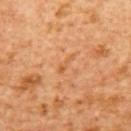{
  "biopsy_status": "not biopsied; imaged during a skin examination",
  "patient": {
    "sex": "female",
    "age_approx": 55
  },
  "lesion_size": {
    "long_diameter_mm_approx": 2.5
  },
  "lighting": "cross-polarized",
  "image": {
    "source": "total-body photography crop",
    "field_of_view_mm": 15
  },
  "site": "upper back"
}